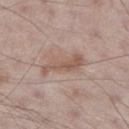{
  "biopsy_status": "not biopsied; imaged during a skin examination",
  "patient": {
    "sex": "male",
    "age_approx": 60
  },
  "site": "leg",
  "image": {
    "source": "total-body photography crop",
    "field_of_view_mm": 15
  }
}